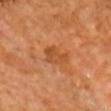The lesion was photographed on a routine skin check and not biopsied; there is no pathology result. A male patient, about 70 years old. This image is a 15 mm lesion crop taken from a total-body photograph. The lesion is on the front of the torso.